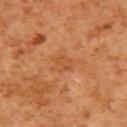<tbp_lesion>
<automated_metrics>
  <eccentricity>0.65</eccentricity>
  <shape_asymmetry>0.25</shape_asymmetry>
  <cielab_L>39</cielab_L>
  <cielab_a>22</cielab_a>
  <cielab_b>32</cielab_b>
  <vs_skin_darker_L>5.0</vs_skin_darker_L>
  <lesion_detection_confidence_0_100>100</lesion_detection_confidence_0_100>
</automated_metrics>
<lighting>cross-polarized</lighting>
<image>
  <source>total-body photography crop</source>
  <field_of_view_mm>15</field_of_view_mm>
</image>
<patient>
  <sex>male</sex>
  <age_approx>80</age_approx>
</patient>
<lesion_size>
  <long_diameter_mm_approx>2.5</long_diameter_mm_approx>
</lesion_size>
<site>upper back</site>
</tbp_lesion>